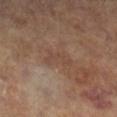follow-up: imaged on a skin check; not biopsied
image-analysis metrics: an average lesion color of about L≈42 a*≈17 b*≈25 (CIELAB), about 5 CIELAB-L* units darker than the surrounding skin, and a normalized lesion–skin contrast near 4.5; a nevus-likeness score of about 0/100 and a lesion-detection confidence of about 95/100
patient: female, aged around 65
imaging modality: 15 mm crop, total-body photography
lesion diameter: about 3.5 mm
body site: the left leg
illumination: cross-polarized illumination